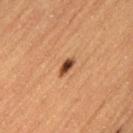Q: Was this lesion biopsied?
A: total-body-photography surveillance lesion; no biopsy
Q: What are the patient's age and sex?
A: female, aged 58 to 62
Q: What is the lesion's diameter?
A: ~2.5 mm (longest diameter)
Q: Automated lesion metrics?
A: a classifier nevus-likeness of about 95/100 and a detector confidence of about 100 out of 100 that the crop contains a lesion
Q: What is the imaging modality?
A: ~15 mm tile from a whole-body skin photo
Q: Lesion location?
A: the left thigh